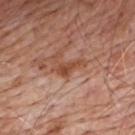Case summary:
- workup — catalogued during a skin exam; not biopsied
- acquisition — ~15 mm tile from a whole-body skin photo
- tile lighting — white-light
- location — the upper back
- automated lesion analysis — a shape eccentricity near 0.9 and a symmetry-axis asymmetry near 0.4; roughly 10 lightness units darker than nearby skin and a lesion-to-skin contrast of about 8.5 (normalized; higher = more distinct); a lesion-detection confidence of about 95/100
- subject — male, about 80 years old